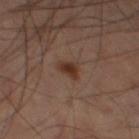workup = catalogued during a skin exam; not biopsied
patient = male, aged around 60
tile lighting = cross-polarized illumination
location = the left thigh
acquisition = total-body-photography crop, ~15 mm field of view